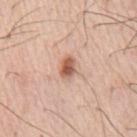biopsy status=catalogued during a skin exam; not biopsied | acquisition=15 mm crop, total-body photography | site=the chest | patient=male, in their mid- to late 70s.